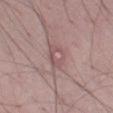• notes — total-body-photography surveillance lesion; no biopsy
• image-analysis metrics — a border-irregularity index near 2/10 and peripheral color asymmetry of about 1.5
• subject — male, roughly 25 years of age
• body site — the leg
• imaging modality — ~15 mm tile from a whole-body skin photo
• lesion diameter — about 3 mm
• tile lighting — white-light illumination